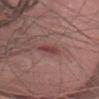biopsy status: catalogued during a skin exam; not biopsied
lighting: white-light illumination
subject: female, aged approximately 50
TBP lesion metrics: a border-irregularity index near 3/10 and a color-variation rating of about 0/10; a nevus-likeness score of about 25/100 and a lesion-detection confidence of about 80/100
site: the head or neck
imaging modality: ~15 mm tile from a whole-body skin photo
size: ~3 mm (longest diameter)